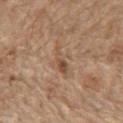Assessment:
Imaged during a routine full-body skin examination; the lesion was not biopsied and no histopathology is available.
Clinical summary:
Automated image analysis of the tile measured an automated nevus-likeness rating near 0 out of 100 and lesion-presence confidence of about 75/100. On the chest. Approximately 5 mm at its widest. The subject is a male aged around 70. Imaged with white-light lighting. A 15 mm crop from a total-body photograph taken for skin-cancer surveillance.